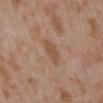image source = 15 mm crop, total-body photography | anatomic site = the upper back | illumination = white-light | lesion diameter = about 3.5 mm | image-analysis metrics = an average lesion color of about L≈51 a*≈18 b*≈31 (CIELAB), about 7 CIELAB-L* units darker than the surrounding skin, and a normalized lesion–skin contrast near 6.5; a border-irregularity index near 3/10, a color-variation rating of about 1.5/10, and radial color variation of about 0.5 | patient = female, in their mid-30s.